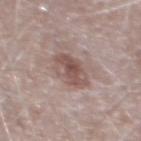The lesion was tiled from a total-body skin photograph and was not biopsied.
On the chest.
The tile uses white-light illumination.
Approximately 3.5 mm at its widest.
A roughly 15 mm field-of-view crop from a total-body skin photograph.
The subject is a male in their mid- to late 70s.